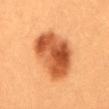{"biopsy_status": "not biopsied; imaged during a skin examination", "image": {"source": "total-body photography crop", "field_of_view_mm": 15}, "patient": {"sex": "female", "age_approx": 30}, "lighting": "cross-polarized", "site": "mid back"}